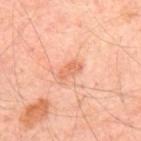workup: catalogued during a skin exam; not biopsied
image source: 15 mm crop, total-body photography
subject: aged 53–57
tile lighting: cross-polarized
lesion size: about 3 mm
site: the upper back